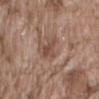Findings:
– follow-up — total-body-photography surveillance lesion; no biopsy
– automated lesion analysis — an outline eccentricity of about 0.8 (0 = round, 1 = elongated); an average lesion color of about L≈47 a*≈19 b*≈27 (CIELAB), about 8 CIELAB-L* units darker than the surrounding skin, and a lesion-to-skin contrast of about 6.5 (normalized; higher = more distinct); a border-irregularity rating of about 4.5/10, a within-lesion color-variation index near 3/10, and radial color variation of about 1
– lesion diameter — ~2.5 mm (longest diameter)
– illumination — white-light illumination
– image source — total-body-photography crop, ~15 mm field of view
– site — the back
– patient — male, about 75 years old